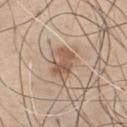{
  "image": {
    "source": "total-body photography crop",
    "field_of_view_mm": 15
  },
  "patient": {
    "sex": "male",
    "age_approx": 45
  },
  "lighting": "white-light",
  "site": "chest",
  "automated_metrics": {
    "border_irregularity_0_10": 2.0,
    "color_variation_0_10": 5.5,
    "peripheral_color_asymmetry": 2.0,
    "nevus_likeness_0_100": 90,
    "lesion_detection_confidence_0_100": 100
  },
  "lesion_size": {
    "long_diameter_mm_approx": 4.0
  }
}